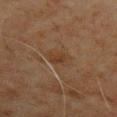Clinical impression:
The lesion was photographed on a routine skin check and not biopsied; there is no pathology result.
Background:
Imaged with cross-polarized lighting. About 2.5 mm across. The lesion-visualizer software estimated roughly 5 lightness units darker than nearby skin and a normalized lesion–skin contrast near 6. The software also gave a border-irregularity index near 2.5/10, a within-lesion color-variation index near 2/10, and peripheral color asymmetry of about 0.5. The software also gave a classifier nevus-likeness of about 10/100 and lesion-presence confidence of about 95/100. The lesion is located on the right upper arm. A 15 mm close-up tile from a total-body photography series done for melanoma screening. The subject is a male approximately 70 years of age.